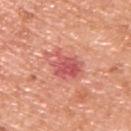Q: Was this lesion biopsied?
A: imaged on a skin check; not biopsied
Q: Who is the patient?
A: male, in their 50s
Q: What lighting was used for the tile?
A: white-light illumination
Q: How was this image acquired?
A: 15 mm crop, total-body photography
Q: What is the anatomic site?
A: the back
Q: How large is the lesion?
A: ≈5 mm